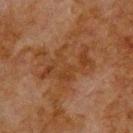<case>
<biopsy_status>not biopsied; imaged during a skin examination</biopsy_status>
<lighting>cross-polarized</lighting>
<lesion_size>
  <long_diameter_mm_approx>7.0</long_diameter_mm_approx>
</lesion_size>
<site>upper back</site>
<patient>
  <sex>male</sex>
  <age_approx>80</age_approx>
</patient>
<image>
  <source>total-body photography crop</source>
  <field_of_view_mm>15</field_of_view_mm>
</image>
<automated_metrics>
  <area_mm2_approx>16.0</area_mm2_approx>
  <eccentricity>0.8</eccentricity>
  <shape_asymmetry>0.55</shape_asymmetry>
  <border_irregularity_0_10>9.0</border_irregularity_0_10>
  <color_variation_0_10>3.5</color_variation_0_10>
</automated_metrics>
</case>